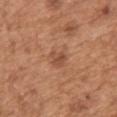Part of a total-body skin-imaging series; this lesion was reviewed on a skin check and was not flagged for biopsy. Automated image analysis of the tile measured border irregularity of about 3.5 on a 0–10 scale, internal color variation of about 2 on a 0–10 scale, and peripheral color asymmetry of about 0.5. A male patient approximately 65 years of age. This is a white-light tile. Cropped from a whole-body photographic skin survey; the tile spans about 15 mm. The lesion is located on the upper back. Measured at roughly 2.5 mm in maximum diameter.